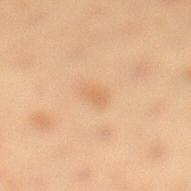Assessment:
Imaged during a routine full-body skin examination; the lesion was not biopsied and no histopathology is available.
Clinical summary:
Longest diameter approximately 2.5 mm. On the left lower leg. This image is a 15 mm lesion crop taken from a total-body photograph. The subject is a female aged approximately 40.